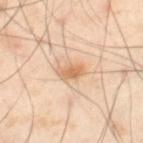Assessment:
Imaged during a routine full-body skin examination; the lesion was not biopsied and no histopathology is available.
Background:
Captured under cross-polarized illumination. Automated image analysis of the tile measured a footprint of about 4.5 mm² and a symmetry-axis asymmetry near 0.2. And it measured a border-irregularity index near 2.5/10, internal color variation of about 2.5 on a 0–10 scale, and a peripheral color-asymmetry measure near 0.5. Cropped from a whole-body photographic skin survey; the tile spans about 15 mm. A male subject, in their mid-50s. The lesion is located on the left thigh. About 3 mm across.